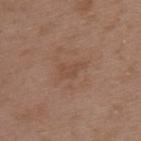  biopsy_status: not biopsied; imaged during a skin examination
  patient:
    sex: male
    age_approx: 50
  image:
    source: total-body photography crop
    field_of_view_mm: 15
  site: upper back
  automated_metrics:
    cielab_L: 47
    cielab_a: 18
    cielab_b: 29
    vs_skin_darker_L: 5.0
    vs_skin_contrast_norm: 4.5
    border_irregularity_0_10: 5.0
    color_variation_0_10: 1.5
    peripheral_color_asymmetry: 0.5
    nevus_likeness_0_100: 0
    lesion_detection_confidence_0_100: 100